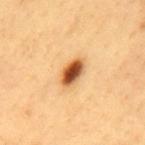Case summary:
– workup: imaged on a skin check; not biopsied
– site: the mid back
– TBP lesion metrics: a lesion area of about 6 mm² and a shape-asymmetry score of about 0.2 (0 = symmetric); an automated nevus-likeness rating near 100 out of 100 and lesion-presence confidence of about 100/100
– patient: male, about 60 years old
– acquisition: ~15 mm crop, total-body skin-cancer survey